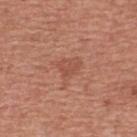  biopsy_status: not biopsied; imaged during a skin examination
  patient:
    sex: male
    age_approx: 45
  site: chest
  image:
    source: total-body photography crop
    field_of_view_mm: 15
  lighting: white-light